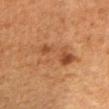notes: imaged on a skin check; not biopsied
patient: female, aged approximately 40
image-analysis metrics: a footprint of about 14 mm², an outline eccentricity of about 0.7 (0 = round, 1 = elongated), and a shape-asymmetry score of about 0.3 (0 = symmetric); an average lesion color of about L≈42 a*≈20 b*≈32 (CIELAB), roughly 6 lightness units darker than nearby skin, and a lesion-to-skin contrast of about 5.5 (normalized; higher = more distinct); border irregularity of about 4.5 on a 0–10 scale, a color-variation rating of about 6.5/10, and peripheral color asymmetry of about 2; a classifier nevus-likeness of about 10/100 and a lesion-detection confidence of about 100/100
diameter: ~5.5 mm (longest diameter)
acquisition: ~15 mm tile from a whole-body skin photo
anatomic site: the right upper arm